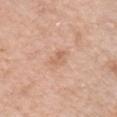follow-up = catalogued during a skin exam; not biopsied
patient = female, in their mid- to late 60s
body site = the back
lesion diameter = about 2.5 mm
illumination = white-light illumination
acquisition = 15 mm crop, total-body photography